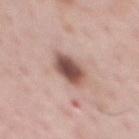The lesion was photographed on a routine skin check and not biopsied; there is no pathology result. The lesion-visualizer software estimated an area of roughly 8.5 mm². The analysis additionally found a lesion color around L≈51 a*≈21 b*≈23 in CIELAB and a lesion-to-skin contrast of about 11.5 (normalized; higher = more distinct). It also reported a nevus-likeness score of about 85/100 and a lesion-detection confidence of about 100/100. Measured at roughly 3.5 mm in maximum diameter. On the chest. A male subject, aged around 60. A lesion tile, about 15 mm wide, cut from a 3D total-body photograph.